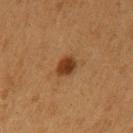<tbp_lesion>
<biopsy_status>not biopsied; imaged during a skin examination</biopsy_status>
<site>left upper arm</site>
<image>
  <source>total-body photography crop</source>
  <field_of_view_mm>15</field_of_view_mm>
</image>
<patient>
  <sex>male</sex>
  <age_approx>55</age_approx>
</patient>
<lighting>cross-polarized</lighting>
<lesion_size>
  <long_diameter_mm_approx>2.5</long_diameter_mm_approx>
</lesion_size>
</tbp_lesion>